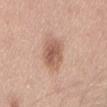Recorded during total-body skin imaging; not selected for excision or biopsy. The lesion is on the mid back. The subject is a male in their 30s. This image is a 15 mm lesion crop taken from a total-body photograph. The lesion's longest dimension is about 4.5 mm. Captured under white-light illumination. Automated image analysis of the tile measured a lesion area of about 10 mm², a shape eccentricity near 0.85, and a shape-asymmetry score of about 0.1 (0 = symmetric). The analysis additionally found a mean CIELAB color near L≈58 a*≈21 b*≈29 and a lesion–skin lightness drop of about 12. It also reported an automated nevus-likeness rating near 65 out of 100 and a detector confidence of about 100 out of 100 that the crop contains a lesion.